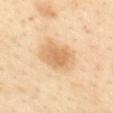{"biopsy_status": "not biopsied; imaged during a skin examination", "lesion_size": {"long_diameter_mm_approx": 4.5}, "lighting": "cross-polarized", "site": "mid back", "patient": {"sex": "female", "age_approx": 40}, "image": {"source": "total-body photography crop", "field_of_view_mm": 15}}